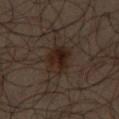Q: Was a biopsy performed?
A: total-body-photography surveillance lesion; no biopsy
Q: Lesion location?
A: the left thigh
Q: What did automated image analysis measure?
A: a lesion color around L≈20 a*≈12 b*≈19 in CIELAB, a lesion–skin lightness drop of about 7, and a normalized border contrast of about 9.5; an automated nevus-likeness rating near 80 out of 100 and a lesion-detection confidence of about 100/100
Q: Lesion size?
A: ~4 mm (longest diameter)
Q: What kind of image is this?
A: total-body-photography crop, ~15 mm field of view
Q: What are the patient's age and sex?
A: male, aged around 65